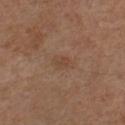Imaged during a routine full-body skin examination; the lesion was not biopsied and no histopathology is available. The recorded lesion diameter is about 3 mm. A close-up tile cropped from a whole-body skin photograph, about 15 mm across. The subject is a male approximately 55 years of age. An algorithmic analysis of the crop reported a lesion color around L≈45 a*≈18 b*≈29 in CIELAB and a normalized lesion–skin contrast near 4.5. The analysis additionally found border irregularity of about 2.5 on a 0–10 scale, a color-variation rating of about 1.5/10, and a peripheral color-asymmetry measure near 0.5. On the chest. This is a white-light tile.